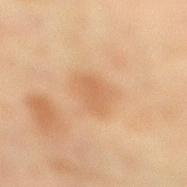Findings:
* follow-up — no biopsy performed (imaged during a skin exam)
* lesion size — ≈3 mm
* location — the right lower leg
* patient — female, about 55 years old
* image source — 15 mm crop, total-body photography
* tile lighting — cross-polarized
* automated metrics — a lesion area of about 5.5 mm² and a shape eccentricity near 0.65; a border-irregularity index near 2/10, a within-lesion color-variation index near 1.5/10, and a peripheral color-asymmetry measure near 0.5; a detector confidence of about 100 out of 100 that the crop contains a lesion